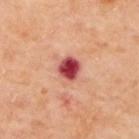workup: total-body-photography surveillance lesion; no biopsy
subject: female, aged approximately 60
automated lesion analysis: a lesion color around L≈49 a*≈36 b*≈26 in CIELAB, about 20 CIELAB-L* units darker than the surrounding skin, and a normalized lesion–skin contrast near 13.5; a nevus-likeness score of about 0/100
tile lighting: cross-polarized illumination
lesion size: ~3 mm (longest diameter)
imaging modality: ~15 mm tile from a whole-body skin photo
site: the upper back